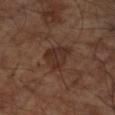– biopsy status — catalogued during a skin exam; not biopsied
– illumination — cross-polarized illumination
– subject — male, aged 63 to 67
– lesion diameter — ≈4 mm
– image — total-body-photography crop, ~15 mm field of view
– body site — the right forearm